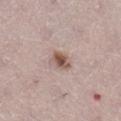Imaged during a routine full-body skin examination; the lesion was not biopsied and no histopathology is available. Automated image analysis of the tile measured an eccentricity of roughly 0.55 and a symmetry-axis asymmetry near 0.25. It also reported an average lesion color of about L≈54 a*≈17 b*≈24 (CIELAB), about 13 CIELAB-L* units darker than the surrounding skin, and a normalized lesion–skin contrast near 9. It also reported a within-lesion color-variation index near 5/10 and radial color variation of about 1.5. And it measured a classifier nevus-likeness of about 95/100 and a detector confidence of about 100 out of 100 that the crop contains a lesion. This image is a 15 mm lesion crop taken from a total-body photograph. Longest diameter approximately 2.5 mm. Located on the right lower leg. A female subject aged approximately 50.